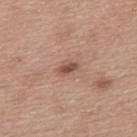Context:
Located on the back. A 15 mm close-up tile from a total-body photography series done for melanoma screening. The lesion-visualizer software estimated a lesion area of about 3.5 mm², an outline eccentricity of about 0.8 (0 = round, 1 = elongated), and a symmetry-axis asymmetry near 0.3. The software also gave a classifier nevus-likeness of about 80/100 and lesion-presence confidence of about 100/100. The recorded lesion diameter is about 2.5 mm. A female subject, in their 40s.